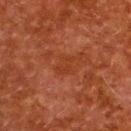| key | value |
|---|---|
| workup | total-body-photography surveillance lesion; no biopsy |
| imaging modality | 15 mm crop, total-body photography |
| subject | female, aged 48–52 |
| automated metrics | a lesion color around L≈32 a*≈26 b*≈31 in CIELAB, roughly 4 lightness units darker than nearby skin, and a lesion-to-skin contrast of about 5 (normalized; higher = more distinct); a nevus-likeness score of about 0/100 and lesion-presence confidence of about 100/100 |
| location | the upper back |
| diameter | about 2.5 mm |
| illumination | cross-polarized illumination |